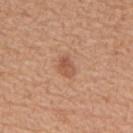Imaged during a routine full-body skin examination; the lesion was not biopsied and no histopathology is available. Located on the upper back. A male subject in their mid- to late 60s. This image is a 15 mm lesion crop taken from a total-body photograph.